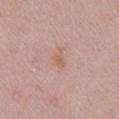acquisition: ~15 mm crop, total-body skin-cancer survey
subject: female, aged 48 to 52
lesion size: ~2.5 mm (longest diameter)
body site: the right upper arm
lighting: white-light illumination